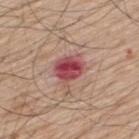Notes:
* workup — total-body-photography surveillance lesion; no biopsy
* illumination — white-light
* TBP lesion metrics — an area of roughly 9.5 mm², an outline eccentricity of about 0.5 (0 = round, 1 = elongated), and a shape-asymmetry score of about 0.25 (0 = symmetric); a color-variation rating of about 8.5/10 and a peripheral color-asymmetry measure near 3
* anatomic site — the upper back
* diameter — ≈3.5 mm
* acquisition — ~15 mm crop, total-body skin-cancer survey
* subject — male, aged 68 to 72